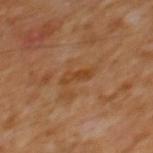No biopsy was performed on this lesion — it was imaged during a full skin examination and was not determined to be concerning.
The lesion-visualizer software estimated an average lesion color of about L≈40 a*≈21 b*≈35 (CIELAB), roughly 7 lightness units darker than nearby skin, and a normalized lesion–skin contrast near 7. It also reported a border-irregularity index near 4/10, internal color variation of about 0 on a 0–10 scale, and peripheral color asymmetry of about 0.
Imaged with cross-polarized lighting.
A close-up tile cropped from a whole-body skin photograph, about 15 mm across.
A male patient aged 63 to 67.
On the mid back.
Measured at roughly 3.5 mm in maximum diameter.